A female subject, aged 58–62. Located on the upper back. A roughly 15 mm field-of-view crop from a total-body skin photograph — 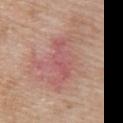automated metrics — a lesion color around L≈55 a*≈27 b*≈23 in CIELAB, a lesion–skin lightness drop of about 7, and a normalized lesion–skin contrast near 5; a border-irregularity rating of about 6/10, internal color variation of about 3 on a 0–10 scale, and peripheral color asymmetry of about 1
tile lighting — white-light
lesion size — ≈5.5 mm
histopathologic diagnosis — a superficial basal cell carcinoma (malignant)